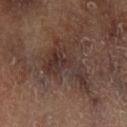Impression:
No biopsy was performed on this lesion — it was imaged during a full skin examination and was not determined to be concerning.
Context:
About 7 mm across. A region of skin cropped from a whole-body photographic capture, roughly 15 mm wide. A male patient, aged 63 to 67. The lesion is on the left lower leg. Automated image analysis of the tile measured an area of roughly 15 mm², an eccentricity of roughly 0.75, and a symmetry-axis asymmetry near 0.65. And it measured a border-irregularity rating of about 9.5/10, a within-lesion color-variation index near 4.5/10, and a peripheral color-asymmetry measure near 1.5. And it measured a lesion-detection confidence of about 90/100. The tile uses cross-polarized illumination.